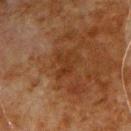Recorded during total-body skin imaging; not selected for excision or biopsy.